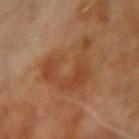Captured during whole-body skin photography for melanoma surveillance; the lesion was not biopsied.
The total-body-photography lesion software estimated an area of roughly 18 mm², a shape eccentricity near 0.7, and a shape-asymmetry score of about 0.65 (0 = symmetric). The software also gave an average lesion color of about L≈35 a*≈19 b*≈29 (CIELAB) and about 6 CIELAB-L* units darker than the surrounding skin. And it measured border irregularity of about 10 on a 0–10 scale. The software also gave a nevus-likeness score of about 0/100.
A 15 mm crop from a total-body photograph taken for skin-cancer surveillance.
The lesion is located on the right upper arm.
A male subject aged approximately 70.
Measured at roughly 6.5 mm in maximum diameter.
The tile uses cross-polarized illumination.